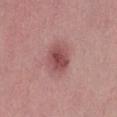{
  "biopsy_status": "not biopsied; imaged during a skin examination",
  "lighting": "white-light",
  "automated_metrics": {
    "eccentricity": 0.55
  },
  "image": {
    "source": "total-body photography crop",
    "field_of_view_mm": 15
  },
  "lesion_size": {
    "long_diameter_mm_approx": 4.0
  },
  "patient": {
    "sex": "female",
    "age_approx": 45
  },
  "site": "leg"
}